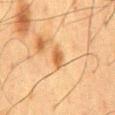Clinical impression:
This lesion was catalogued during total-body skin photography and was not selected for biopsy.
Acquisition and patient details:
A male subject, aged 58–62. The lesion is located on the back. Cropped from a whole-body photographic skin survey; the tile spans about 15 mm.